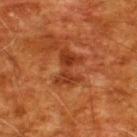automated_metrics:
  cielab_L: 36
  cielab_a: 29
  cielab_b: 36
  vs_skin_darker_L: 9.0
  vs_skin_contrast_norm: 7.5
  border_irregularity_0_10: 6.5
  peripheral_color_asymmetry: 1.0
  nevus_likeness_0_100: 0
  lesion_detection_confidence_0_100: 100
site: upper back
patient:
  sex: male
  age_approx: 60
lighting: cross-polarized
lesion_size:
  long_diameter_mm_approx: 4.0
image:
  source: total-body photography crop
  field_of_view_mm: 15